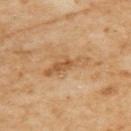{
  "biopsy_status": "not biopsied; imaged during a skin examination"
}